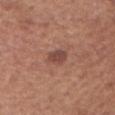Impression: Recorded during total-body skin imaging; not selected for excision or biopsy. Image and clinical context: The lesion's longest dimension is about 2.5 mm. A 15 mm close-up tile from a total-body photography series done for melanoma screening. This is a white-light tile. Automated image analysis of the tile measured a border-irregularity index near 2/10, a within-lesion color-variation index near 2/10, and radial color variation of about 0.5. The software also gave a nevus-likeness score of about 80/100. On the head or neck. A male subject, aged approximately 65.